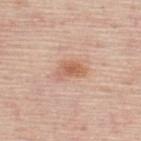notes: catalogued during a skin exam; not biopsied | image: ~15 mm tile from a whole-body skin photo | lesion size: ≈3.5 mm | image-analysis metrics: an area of roughly 6 mm², an outline eccentricity of about 0.8 (0 = round, 1 = elongated), and two-axis asymmetry of about 0.4; a border-irregularity rating of about 3.5/10, a color-variation rating of about 3.5/10, and a peripheral color-asymmetry measure near 1; a classifier nevus-likeness of about 80/100 | illumination: white-light | subject: male, aged around 45 | location: the upper back.